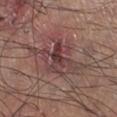{"biopsy_status": "not biopsied; imaged during a skin examination", "image": {"source": "total-body photography crop", "field_of_view_mm": 15}, "site": "right lower leg", "patient": {"sex": "male", "age_approx": 45}}